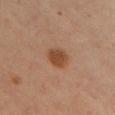{"site": "chest", "automated_metrics": {"area_mm2_approx": 6.0, "cielab_L": 45, "cielab_a": 21, "cielab_b": 33, "vs_skin_darker_L": 10.0, "vs_skin_contrast_norm": 9.0}, "lesion_size": {"long_diameter_mm_approx": 3.0}, "image": {"source": "total-body photography crop", "field_of_view_mm": 15}, "lighting": "cross-polarized", "patient": {"sex": "female", "age_approx": 40}}